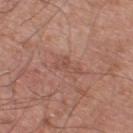Q: Was this lesion biopsied?
A: catalogued during a skin exam; not biopsied
Q: Lesion location?
A: the back
Q: How large is the lesion?
A: about 3.5 mm
Q: What are the patient's age and sex?
A: male, in their mid- to late 50s
Q: What kind of image is this?
A: 15 mm crop, total-body photography
Q: What lighting was used for the tile?
A: white-light